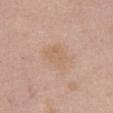Clinical impression:
This lesion was catalogued during total-body skin photography and was not selected for biopsy.
Background:
About 4 mm across. On the left thigh. A female patient roughly 50 years of age. Automated tile analysis of the lesion measured a nevus-likeness score of about 0/100 and lesion-presence confidence of about 100/100. The tile uses white-light illumination. Cropped from a whole-body photographic skin survey; the tile spans about 15 mm.